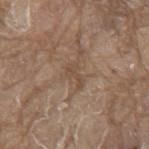The lesion was photographed on a routine skin check and not biopsied; there is no pathology result. The total-body-photography lesion software estimated an outline eccentricity of about 0.85 (0 = round, 1 = elongated) and a symmetry-axis asymmetry near 0.6. The software also gave roughly 7 lightness units darker than nearby skin and a normalized border contrast of about 5.5. It also reported border irregularity of about 7 on a 0–10 scale and a within-lesion color-variation index near 0/10. Longest diameter approximately 3 mm. The patient is a male approximately 80 years of age. The tile uses white-light illumination. A roughly 15 mm field-of-view crop from a total-body skin photograph. Located on the mid back.